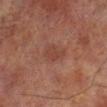Recorded during total-body skin imaging; not selected for excision or biopsy. A 15 mm close-up tile from a total-body photography series done for melanoma screening. On the left lower leg. A male subject aged 68–72.